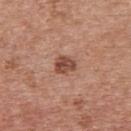Impression:
The lesion was tiled from a total-body skin photograph and was not biopsied.
Acquisition and patient details:
Located on the upper back. Captured under white-light illumination. A female subject aged 38–42. A roughly 15 mm field-of-view crop from a total-body skin photograph. Longest diameter approximately 2.5 mm.